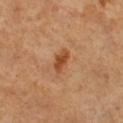| feature | finding |
|---|---|
| notes | total-body-photography surveillance lesion; no biopsy |
| diameter | about 3 mm |
| automated metrics | an area of roughly 4 mm², an eccentricity of roughly 0.85, and a symmetry-axis asymmetry near 0.25; an automated nevus-likeness rating near 75 out of 100 |
| image source | total-body-photography crop, ~15 mm field of view |
| body site | the right lower leg |
| subject | female, roughly 55 years of age |
| lighting | cross-polarized |